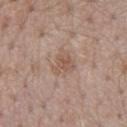<lesion>
  <biopsy_status>not biopsied; imaged during a skin examination</biopsy_status>
  <image>
    <source>total-body photography crop</source>
    <field_of_view_mm>15</field_of_view_mm>
  </image>
  <site>mid back</site>
  <patient>
    <sex>male</sex>
    <age_approx>55</age_approx>
  </patient>
</lesion>